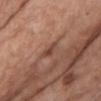notes: catalogued during a skin exam; not biopsied